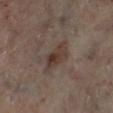| key | value |
|---|---|
| follow-up | catalogued during a skin exam; not biopsied |
| lighting | cross-polarized |
| patient | female, aged approximately 60 |
| lesion diameter | about 4 mm |
| site | the leg |
| image | ~15 mm tile from a whole-body skin photo |